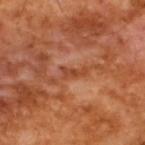{
  "biopsy_status": "not biopsied; imaged during a skin examination",
  "lighting": "cross-polarized",
  "image": {
    "source": "total-body photography crop",
    "field_of_view_mm": 15
  },
  "lesion_size": {
    "long_diameter_mm_approx": 3.0
  },
  "automated_metrics": {
    "eccentricity": 0.9,
    "cielab_L": 44,
    "cielab_a": 28,
    "cielab_b": 36,
    "vs_skin_darker_L": 8.0,
    "vs_skin_contrast_norm": 6.5,
    "border_irregularity_0_10": 5.5,
    "color_variation_0_10": 0.0,
    "peripheral_color_asymmetry": 0.0,
    "nevus_likeness_0_100": 0
  },
  "patient": {
    "sex": "male",
    "age_approx": 65
  }
}